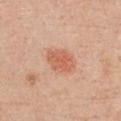Clinical impression: Part of a total-body skin-imaging series; this lesion was reviewed on a skin check and was not flagged for biopsy. Acquisition and patient details: An algorithmic analysis of the crop reported a border-irregularity rating of about 2/10 and internal color variation of about 2 on a 0–10 scale. Cropped from a whole-body photographic skin survey; the tile spans about 15 mm. The tile uses white-light illumination. Measured at roughly 3.5 mm in maximum diameter. A female patient aged around 40. The lesion is on the chest.